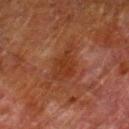Clinical impression:
No biopsy was performed on this lesion — it was imaged during a full skin examination and was not determined to be concerning.
Clinical summary:
The recorded lesion diameter is about 5.5 mm. Located on the right lower leg. A 15 mm close-up tile from a total-body photography series done for melanoma screening. Imaged with cross-polarized lighting. The patient is a male in their 80s. Automated tile analysis of the lesion measured a lesion area of about 8.5 mm², an outline eccentricity of about 0.9 (0 = round, 1 = elongated), and a shape-asymmetry score of about 0.4 (0 = symmetric). And it measured border irregularity of about 5 on a 0–10 scale, internal color variation of about 1.5 on a 0–10 scale, and a peripheral color-asymmetry measure near 0.5. The analysis additionally found a classifier nevus-likeness of about 0/100 and a detector confidence of about 100 out of 100 that the crop contains a lesion.